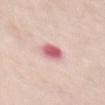<case>
<lesion_size>
  <long_diameter_mm_approx>3.0</long_diameter_mm_approx>
</lesion_size>
<lighting>white-light</lighting>
<image>
  <source>total-body photography crop</source>
  <field_of_view_mm>15</field_of_view_mm>
</image>
<site>abdomen</site>
<patient>
  <sex>female</sex>
  <age_approx>70</age_approx>
</patient>
</case>